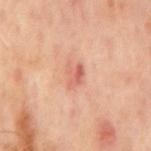The lesion was tiled from a total-body skin photograph and was not biopsied. Cropped from a total-body skin-imaging series; the visible field is about 15 mm. A male subject, aged around 65. On the mid back. Approximately 2.5 mm at its widest.